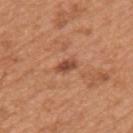notes = no biopsy performed (imaged during a skin exam); lesion size = about 3 mm; image = total-body-photography crop, ~15 mm field of view; subject = male, aged approximately 65; site = the left upper arm.